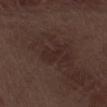Assessment: Imaged during a routine full-body skin examination; the lesion was not biopsied and no histopathology is available. Context: The recorded lesion diameter is about 3 mm. The lesion is on the right thigh. A lesion tile, about 15 mm wide, cut from a 3D total-body photograph. This is a white-light tile. The subject is a male aged 68–72.